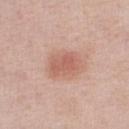Recorded during total-body skin imaging; not selected for excision or biopsy. A 15 mm close-up extracted from a 3D total-body photography capture. The subject is a female approximately 60 years of age. The lesion is on the right lower leg. The total-body-photography lesion software estimated a lesion area of about 9.5 mm², an eccentricity of roughly 0.75, and a symmetry-axis asymmetry near 0.15. The analysis additionally found a lesion color around L≈60 a*≈24 b*≈28 in CIELAB, about 9 CIELAB-L* units darker than the surrounding skin, and a normalized lesion–skin contrast near 6. The analysis additionally found border irregularity of about 2 on a 0–10 scale, internal color variation of about 2.5 on a 0–10 scale, and radial color variation of about 1. The analysis additionally found a nevus-likeness score of about 90/100 and a detector confidence of about 100 out of 100 that the crop contains a lesion. Longest diameter approximately 4 mm.